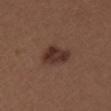Longest diameter approximately 4 mm. The lesion is located on the left upper arm. A male patient aged 28–32. The tile uses white-light illumination. Cropped from a whole-body photographic skin survey; the tile spans about 15 mm.